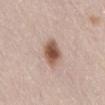| field | value |
|---|---|
| biopsy status | no biopsy performed (imaged during a skin exam) |
| image source | ~15 mm tile from a whole-body skin photo |
| image-analysis metrics | a footprint of about 8 mm², an outline eccentricity of about 0.8 (0 = round, 1 = elongated), and two-axis asymmetry of about 0.1; a classifier nevus-likeness of about 100/100 |
| subject | female, about 65 years old |
| anatomic site | the lower back |